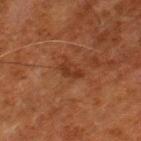The lesion was tiled from a total-body skin photograph and was not biopsied.
An algorithmic analysis of the crop reported a lesion color around L≈27 a*≈20 b*≈26 in CIELAB, roughly 6 lightness units darker than nearby skin, and a normalized border contrast of about 6.5.
A male patient aged 78–82.
The recorded lesion diameter is about 2.5 mm.
A lesion tile, about 15 mm wide, cut from a 3D total-body photograph.
The lesion is located on the left thigh.